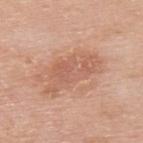Impression:
Imaged during a routine full-body skin examination; the lesion was not biopsied and no histopathology is available.
Acquisition and patient details:
Located on the upper back. The patient is a male aged 78 to 82. Imaged with white-light lighting. Measured at roughly 6.5 mm in maximum diameter. A 15 mm crop from a total-body photograph taken for skin-cancer surveillance.